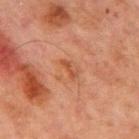| key | value |
|---|---|
| subject | male, approximately 65 years of age |
| automated metrics | a footprint of about 2 mm² and a symmetry-axis asymmetry near 0.35; an average lesion color of about L≈41 a*≈23 b*≈31 (CIELAB), about 7 CIELAB-L* units darker than the surrounding skin, and a lesion-to-skin contrast of about 6.5 (normalized; higher = more distinct); a classifier nevus-likeness of about 0/100 and lesion-presence confidence of about 100/100 |
| lighting | cross-polarized |
| lesion size | about 2.5 mm |
| site | the chest |
| acquisition | 15 mm crop, total-body photography |